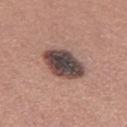workup: imaged on a skin check; not biopsied | anatomic site: the left thigh | diameter: about 5 mm | illumination: white-light | image: 15 mm crop, total-body photography | image-analysis metrics: a lesion area of about 15 mm², an eccentricity of roughly 0.75, and a shape-asymmetry score of about 0.15 (0 = symmetric); an average lesion color of about L≈44 a*≈16 b*≈18 (CIELAB) and a normalized border contrast of about 14; internal color variation of about 7.5 on a 0–10 scale and a peripheral color-asymmetry measure near 2 | subject: female, aged 28–32.